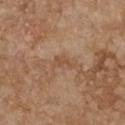The lesion was photographed on a routine skin check and not biopsied; there is no pathology result.
A female patient, aged 63 to 67.
Cropped from a whole-body photographic skin survey; the tile spans about 15 mm.
The lesion is located on the chest.
Captured under white-light illumination.
The lesion's longest dimension is about 2.5 mm.